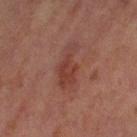body site: the left thigh | diameter: ~5.5 mm (longest diameter) | lighting: cross-polarized | subject: female, approximately 60 years of age | acquisition: 15 mm crop, total-body photography.